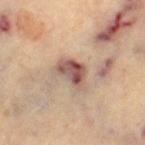biopsy status=no biopsy performed (imaged during a skin exam)
lighting=cross-polarized illumination
anatomic site=the left thigh
diameter=about 3.5 mm
imaging modality=~15 mm crop, total-body skin-cancer survey
patient=female, aged 63–67